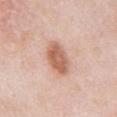The lesion is located on the abdomen. A male subject about 55 years old. Imaged with white-light lighting. A region of skin cropped from a whole-body photographic capture, roughly 15 mm wide. Longest diameter approximately 4.5 mm. Automated tile analysis of the lesion measured a lesion area of about 10 mm² and a symmetry-axis asymmetry near 0.1. It also reported a classifier nevus-likeness of about 95/100 and a lesion-detection confidence of about 100/100.